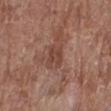| feature | finding |
|---|---|
| follow-up | no biopsy performed (imaged during a skin exam) |
| illumination | white-light illumination |
| size | ≈3.5 mm |
| automated lesion analysis | a lesion area of about 6.5 mm², an outline eccentricity of about 0.7 (0 = round, 1 = elongated), and two-axis asymmetry of about 0.3; a mean CIELAB color near L≈44 a*≈21 b*≈26; a peripheral color-asymmetry measure near 1; a nevus-likeness score of about 0/100 and a detector confidence of about 100 out of 100 that the crop contains a lesion |
| patient | female, in their 80s |
| imaging modality | total-body-photography crop, ~15 mm field of view |
| site | the right lower leg |